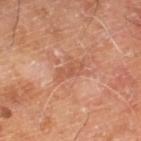Clinical impression: The lesion was tiled from a total-body skin photograph and was not biopsied. Context: The tile uses cross-polarized illumination. A male subject in their mid- to late 60s. A region of skin cropped from a whole-body photographic capture, roughly 15 mm wide. About 3 mm across. On the right lower leg.